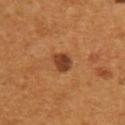Clinical impression:
This lesion was catalogued during total-body skin photography and was not selected for biopsy.
Background:
A lesion tile, about 15 mm wide, cut from a 3D total-body photograph. Automated image analysis of the tile measured a lesion area of about 5 mm², an eccentricity of roughly 0.5, and a symmetry-axis asymmetry near 0.2. The analysis additionally found a lesion color around L≈37 a*≈22 b*≈32 in CIELAB, a lesion–skin lightness drop of about 12, and a normalized lesion–skin contrast near 10. A male patient, aged around 55. On the back.